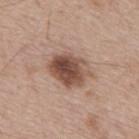{
  "biopsy_status": "not biopsied; imaged during a skin examination",
  "automated_metrics": {
    "eccentricity": 0.65,
    "shape_asymmetry": 0.15,
    "nevus_likeness_0_100": 85
  },
  "image": {
    "source": "total-body photography crop",
    "field_of_view_mm": 15
  },
  "lesion_size": {
    "long_diameter_mm_approx": 5.0
  },
  "patient": {
    "sex": "male",
    "age_approx": 55
  }
}